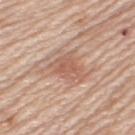Q: Was this lesion biopsied?
A: catalogued during a skin exam; not biopsied
Q: What lighting was used for the tile?
A: white-light
Q: Lesion location?
A: the left upper arm
Q: What kind of image is this?
A: ~15 mm tile from a whole-body skin photo
Q: What are the patient's age and sex?
A: female, aged 63–67